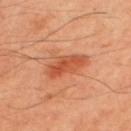Notes:
• notes — catalogued during a skin exam; not biopsied
• automated lesion analysis — a footprint of about 9 mm², an eccentricity of roughly 0.85, and two-axis asymmetry of about 0.25; a border-irregularity index near 3.5/10
• lighting — cross-polarized
• anatomic site — the back
• patient — male, aged 48 to 52
• image source — ~15 mm crop, total-body skin-cancer survey